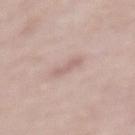The lesion's longest dimension is about 3 mm.
The lesion-visualizer software estimated an average lesion color of about L≈62 a*≈18 b*≈22 (CIELAB) and about 8 CIELAB-L* units darker than the surrounding skin. And it measured lesion-presence confidence of about 95/100.
The tile uses white-light illumination.
On the mid back.
The patient is a female about 65 years old.
This image is a 15 mm lesion crop taken from a total-body photograph.